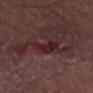This lesion was catalogued during total-body skin photography and was not selected for biopsy. The lesion is on the left thigh. A male patient, roughly 55 years of age. Automated image analysis of the tile measured a classifier nevus-likeness of about 0/100 and a detector confidence of about 90 out of 100 that the crop contains a lesion. Imaged with cross-polarized lighting. A 15 mm crop from a total-body photograph taken for skin-cancer surveillance. Approximately 4 mm at its widest.